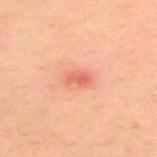  lighting: cross-polarized
  patient:
    sex: male
    age_approx: 40
  site: upper back
  automated_metrics:
    area_mm2_approx: 3.5
    eccentricity: 0.85
    cielab_L: 51
    cielab_a: 28
    cielab_b: 28
    vs_skin_darker_L: 8.0
    vs_skin_contrast_norm: 6.0
    border_irregularity_0_10: 2.0
    color_variation_0_10: 3.5
    nevus_likeness_0_100: 0
    lesion_detection_confidence_0_100: 100
  image:
    source: total-body photography crop
    field_of_view_mm: 15